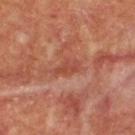Findings:
- follow-up · imaged on a skin check; not biopsied
- automated lesion analysis · an eccentricity of roughly 0.85; a border-irregularity index near 3.5/10, internal color variation of about 2 on a 0–10 scale, and peripheral color asymmetry of about 0.5
- location · the upper back
- diameter · about 3.5 mm
- image source · total-body-photography crop, ~15 mm field of view
- subject · male, about 55 years old